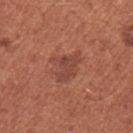The lesion was photographed on a routine skin check and not biopsied; there is no pathology result. The subject is a female aged 33–37. Located on the right upper arm. The tile uses white-light illumination. About 4 mm across. Cropped from a whole-body photographic skin survey; the tile spans about 15 mm.